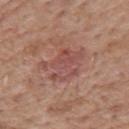automated metrics — a border-irregularity index near 6.5/10, a within-lesion color-variation index near 4/10, and peripheral color asymmetry of about 1.5
patient — male, aged 58–62
image — ~15 mm crop, total-body skin-cancer survey
lesion diameter — ≈5.5 mm
lighting — white-light illumination
location — the mid back